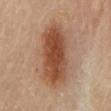notes: catalogued during a skin exam; not biopsied
acquisition: ~15 mm tile from a whole-body skin photo
subject: male, about 85 years old or older
site: the mid back